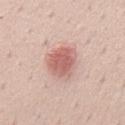The lesion was photographed on a routine skin check and not biopsied; there is no pathology result. A male patient, about 50 years old. The tile uses white-light illumination. A 15 mm close-up extracted from a 3D total-body photography capture. About 4 mm across. Located on the mid back. Automated tile analysis of the lesion measured a lesion area of about 11 mm² and a shape-asymmetry score of about 0.15 (0 = symmetric). The software also gave an average lesion color of about L≈61 a*≈24 b*≈25 (CIELAB), a lesion–skin lightness drop of about 12, and a lesion-to-skin contrast of about 7.5 (normalized; higher = more distinct). The analysis additionally found a classifier nevus-likeness of about 90/100 and a detector confidence of about 100 out of 100 that the crop contains a lesion.